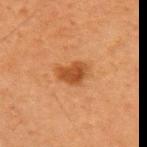* notes · total-body-photography surveillance lesion; no biopsy
* size · ≈3.5 mm
* acquisition · ~15 mm crop, total-body skin-cancer survey
* image-analysis metrics · an area of roughly 6.5 mm² and an outline eccentricity of about 0.75 (0 = round, 1 = elongated); internal color variation of about 2.5 on a 0–10 scale and radial color variation of about 1
* subject · female, aged approximately 60
* body site · the right upper arm
* lighting · cross-polarized